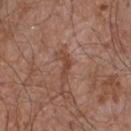The lesion was tiled from a total-body skin photograph and was not biopsied. The lesion-visualizer software estimated an average lesion color of about L≈45 a*≈21 b*≈28 (CIELAB), about 8 CIELAB-L* units darker than the surrounding skin, and a lesion-to-skin contrast of about 6.5 (normalized; higher = more distinct). On the chest. This is a white-light tile. The subject is a male roughly 55 years of age. Measured at roughly 3 mm in maximum diameter. A 15 mm close-up extracted from a 3D total-body photography capture.